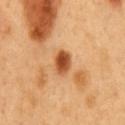Findings:
• workup — no biopsy performed (imaged during a skin exam)
• diameter — about 3 mm
• imaging modality — total-body-photography crop, ~15 mm field of view
• patient — male, aged approximately 50
• TBP lesion metrics — a mean CIELAB color near L≈52 a*≈27 b*≈42, roughly 16 lightness units darker than nearby skin, and a lesion-to-skin contrast of about 10.5 (normalized; higher = more distinct); border irregularity of about 1.5 on a 0–10 scale and internal color variation of about 5 on a 0–10 scale
• anatomic site — the mid back